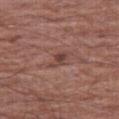Clinical impression:
This lesion was catalogued during total-body skin photography and was not selected for biopsy.
Context:
The patient is a male in their mid-60s. The lesion is located on the right thigh. Measured at roughly 2.5 mm in maximum diameter. This is a white-light tile. A roughly 15 mm field-of-view crop from a total-body skin photograph.